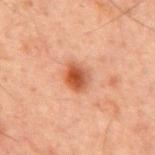Part of a total-body skin-imaging series; this lesion was reviewed on a skin check and was not flagged for biopsy. The subject is a male aged 58 to 62. Imaged with cross-polarized lighting. Approximately 3 mm at its widest. A lesion tile, about 15 mm wide, cut from a 3D total-body photograph. The lesion-visualizer software estimated a lesion color around L≈42 a*≈24 b*≈30 in CIELAB and a normalized lesion–skin contrast near 9.5. The analysis additionally found a border-irregularity index near 1.5/10 and a within-lesion color-variation index near 6/10. The software also gave an automated nevus-likeness rating near 100 out of 100 and a lesion-detection confidence of about 100/100. The lesion is located on the back.